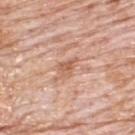{
  "biopsy_status": "not biopsied; imaged during a skin examination",
  "lighting": "white-light",
  "site": "upper back",
  "image": {
    "source": "total-body photography crop",
    "field_of_view_mm": 15
  },
  "patient": {
    "sex": "male",
    "age_approx": 80
  },
  "lesion_size": {
    "long_diameter_mm_approx": 3.0
  }
}